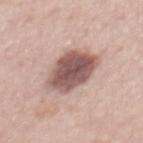Q: Is there a histopathology result?
A: no biopsy performed (imaged during a skin exam)
Q: Patient demographics?
A: male, aged 63–67
Q: Lesion location?
A: the back
Q: What kind of image is this?
A: total-body-photography crop, ~15 mm field of view